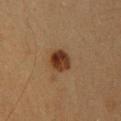{
  "biopsy_status": "not biopsied; imaged during a skin examination",
  "lesion_size": {
    "long_diameter_mm_approx": 3.0
  },
  "automated_metrics": {
    "border_irregularity_0_10": 1.5,
    "color_variation_0_10": 6.0,
    "peripheral_color_asymmetry": 2.0,
    "lesion_detection_confidence_0_100": 100
  },
  "patient": {
    "sex": "female",
    "age_approx": 20
  },
  "site": "upper back",
  "image": {
    "source": "total-body photography crop",
    "field_of_view_mm": 15
  },
  "lighting": "cross-polarized"
}